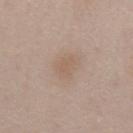Imaged during a routine full-body skin examination; the lesion was not biopsied and no histopathology is available. A male subject about 55 years old. A 15 mm close-up extracted from a 3D total-body photography capture. Located on the front of the torso. This is a white-light tile.